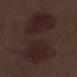<lesion>
<biopsy_status>not biopsied; imaged during a skin examination</biopsy_status>
<automated_metrics>
  <area_mm2_approx>55.0</area_mm2_approx>
  <eccentricity>0.95</eccentricity>
  <shape_asymmetry>0.3</shape_asymmetry>
  <vs_skin_darker_L>6.0</vs_skin_darker_L>
  <vs_skin_contrast_norm>7.0</vs_skin_contrast_norm>
  <border_irregularity_0_10>5.0</border_irregularity_0_10>
  <nevus_likeness_0_100>85</nevus_likeness_0_100>
  <lesion_detection_confidence_0_100>100</lesion_detection_confidence_0_100>
</automated_metrics>
<lighting>white-light</lighting>
<image>
  <source>total-body photography crop</source>
  <field_of_view_mm>15</field_of_view_mm>
</image>
<lesion_size>
  <long_diameter_mm_approx>13.0</long_diameter_mm_approx>
</lesion_size>
<patient>
  <sex>male</sex>
  <age_approx>70</age_approx>
</patient>
<site>leg</site>
</lesion>